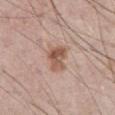Q: Is there a histopathology result?
A: catalogued during a skin exam; not biopsied
Q: Where on the body is the lesion?
A: the abdomen
Q: What is the imaging modality?
A: ~15 mm crop, total-body skin-cancer survey
Q: Patient demographics?
A: male, aged approximately 75